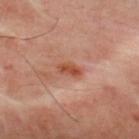The lesion was tiled from a total-body skin photograph and was not biopsied. On the back. A close-up tile cropped from a whole-body skin photograph, about 15 mm across. A male patient about 50 years old.